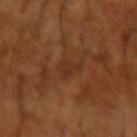workup: imaged on a skin check; not biopsied | subject: male, aged approximately 65 | automated metrics: an average lesion color of about L≈32 a*≈23 b*≈31 (CIELAB) and about 5 CIELAB-L* units darker than the surrounding skin; a nevus-likeness score of about 0/100 | image source: ~15 mm crop, total-body skin-cancer survey | size: ~2.5 mm (longest diameter) | lighting: cross-polarized | site: the right forearm.